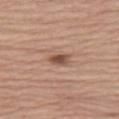| key | value |
|---|---|
| notes | catalogued during a skin exam; not biopsied |
| automated lesion analysis | a footprint of about 3.5 mm²; a mean CIELAB color near L≈50 a*≈21 b*≈27, about 13 CIELAB-L* units darker than the surrounding skin, and a normalized lesion–skin contrast near 9; border irregularity of about 2.5 on a 0–10 scale, a within-lesion color-variation index near 4/10, and radial color variation of about 1.5; a nevus-likeness score of about 90/100 and a detector confidence of about 100 out of 100 that the crop contains a lesion |
| tile lighting | white-light illumination |
| diameter | ~2.5 mm (longest diameter) |
| imaging modality | ~15 mm tile from a whole-body skin photo |
| anatomic site | the leg |
| subject | female, roughly 65 years of age |